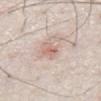The lesion was photographed on a routine skin check and not biopsied; there is no pathology result.
Cropped from a total-body skin-imaging series; the visible field is about 15 mm.
Imaged with white-light lighting.
The subject is a male in their 80s.
From the abdomen.
The recorded lesion diameter is about 2.5 mm.